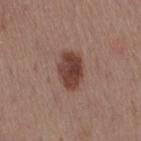* follow-up · no biopsy performed (imaged during a skin exam)
* site · the right thigh
* subject · female, roughly 40 years of age
* imaging modality · ~15 mm tile from a whole-body skin photo
* lesion diameter · ≈4 mm
* tile lighting · white-light illumination
* image-analysis metrics · a classifier nevus-likeness of about 95/100 and lesion-presence confidence of about 100/100